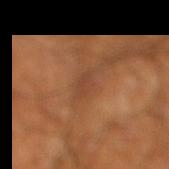Assessment:
Imaged during a routine full-body skin examination; the lesion was not biopsied and no histopathology is available.
Clinical summary:
An algorithmic analysis of the crop reported a border-irregularity index near 2.5/10, a color-variation rating of about 1/10, and radial color variation of about 0.5. The patient is a male aged 58 to 62. A 15 mm crop from a total-body photograph taken for skin-cancer surveillance. Located on the right lower leg. The lesion's longest dimension is about 2.5 mm.